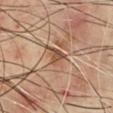{
  "site": "front of the torso",
  "image": {
    "source": "total-body photography crop",
    "field_of_view_mm": 15
  },
  "lighting": "cross-polarized",
  "patient": {
    "sex": "male",
    "age_approx": 70
  },
  "lesion_size": {
    "long_diameter_mm_approx": 3.0
  },
  "automated_metrics": {
    "border_irregularity_0_10": 4.0,
    "color_variation_0_10": 2.5,
    "peripheral_color_asymmetry": 1.0,
    "nevus_likeness_0_100": 0,
    "lesion_detection_confidence_0_100": 60
  }
}